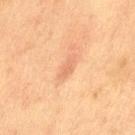Impression: Captured during whole-body skin photography for melanoma surveillance; the lesion was not biopsied. Background: An algorithmic analysis of the crop reported an average lesion color of about L≈59 a*≈22 b*≈33 (CIELAB) and a normalized border contrast of about 5. The software also gave a border-irregularity rating of about 3.5/10, a color-variation rating of about 0.5/10, and a peripheral color-asymmetry measure near 0. The software also gave a nevus-likeness score of about 0/100. A 15 mm close-up extracted from a 3D total-body photography capture. Longest diameter approximately 3 mm. Captured under cross-polarized illumination. On the left thigh. A female patient about 40 years old.